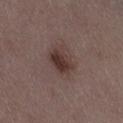{
  "biopsy_status": "not biopsied; imaged during a skin examination",
  "site": "lower back",
  "patient": {
    "sex": "female",
    "age_approx": 35
  },
  "image": {
    "source": "total-body photography crop",
    "field_of_view_mm": 15
  },
  "lighting": "white-light"
}